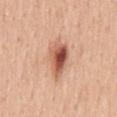Measured at roughly 4.5 mm in maximum diameter.
From the back.
The tile uses white-light illumination.
The patient is a male aged 48 to 52.
A 15 mm crop from a total-body photograph taken for skin-cancer surveillance.